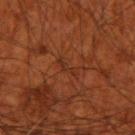Recorded during total-body skin imaging; not selected for excision or biopsy.